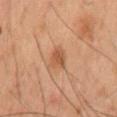Cropped from a whole-body photographic skin survey; the tile spans about 15 mm.
The lesion is located on the mid back.
A male patient, aged approximately 60.
Longest diameter approximately 3 mm.